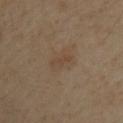Context:
The subject is a male aged 48 to 52. From the arm. Imaged with cross-polarized lighting. A 15 mm close-up extracted from a 3D total-body photography capture.